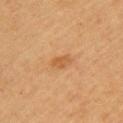{"biopsy_status": "not biopsied; imaged during a skin examination", "image": {"source": "total-body photography crop", "field_of_view_mm": 15}, "site": "left upper arm", "lighting": "cross-polarized", "patient": {"sex": "female", "age_approx": 60}, "lesion_size": {"long_diameter_mm_approx": 2.5}, "automated_metrics": {"eccentricity": 0.8, "shape_asymmetry": 0.35, "cielab_L": 50, "cielab_a": 21, "cielab_b": 38, "vs_skin_darker_L": 7.0}}